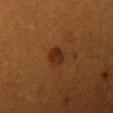Findings:
– biopsy status — no biopsy performed (imaged during a skin exam)
– size — ~2.5 mm (longest diameter)
– image source — total-body-photography crop, ~15 mm field of view
– lighting — cross-polarized
– anatomic site — the right upper arm
– patient — female, about 55 years old